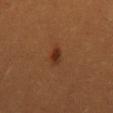Findings:
* size: ~2.5 mm (longest diameter)
* patient: female, aged approximately 30
* acquisition: ~15 mm tile from a whole-body skin photo
* site: the mid back